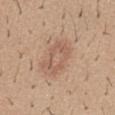Imaged during a routine full-body skin examination; the lesion was not biopsied and no histopathology is available. Cropped from a whole-body photographic skin survey; the tile spans about 15 mm. A male subject, in their 40s. Imaged with white-light lighting. From the abdomen. Approximately 4.5 mm at its widest.